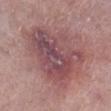Impression:
Captured during whole-body skin photography for melanoma surveillance; the lesion was not biopsied.
Image and clinical context:
Cropped from a whole-body photographic skin survey; the tile spans about 15 mm. On the right lower leg. A female patient, aged 58–62. An algorithmic analysis of the crop reported a lesion area of about 40 mm², an eccentricity of roughly 0.65, and a shape-asymmetry score of about 0.3 (0 = symmetric). It also reported a lesion color around L≈49 a*≈23 b*≈17 in CIELAB, a lesion–skin lightness drop of about 10, and a lesion-to-skin contrast of about 8 (normalized; higher = more distinct). It also reported a border-irregularity rating of about 3.5/10 and a peripheral color-asymmetry measure near 2.5. The tile uses white-light illumination.